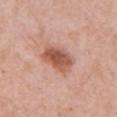No biopsy was performed on this lesion — it was imaged during a full skin examination and was not determined to be concerning. A female patient, approximately 60 years of age. The lesion is on the chest. A 15 mm close-up tile from a total-body photography series done for melanoma screening.